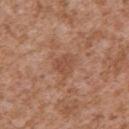{
  "site": "left upper arm",
  "automated_metrics": {
    "area_mm2_approx": 5.0,
    "eccentricity": 0.4,
    "shape_asymmetry": 0.3,
    "cielab_L": 49,
    "cielab_a": 23,
    "cielab_b": 31,
    "vs_skin_darker_L": 7.0,
    "vs_skin_contrast_norm": 5.5,
    "color_variation_0_10": 2.0,
    "nevus_likeness_0_100": 0,
    "lesion_detection_confidence_0_100": 100
  },
  "lighting": "white-light",
  "lesion_size": {
    "long_diameter_mm_approx": 3.0
  },
  "patient": {
    "sex": "male",
    "age_approx": 45
  },
  "image": {
    "source": "total-body photography crop",
    "field_of_view_mm": 15
  }
}